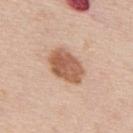Imaged during a routine full-body skin examination; the lesion was not biopsied and no histopathology is available.
Located on the mid back.
A male patient, aged 58–62.
The tile uses white-light illumination.
Cropped from a whole-body photographic skin survey; the tile spans about 15 mm.